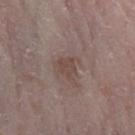Assessment:
Imaged during a routine full-body skin examination; the lesion was not biopsied and no histopathology is available.
Image and clinical context:
An algorithmic analysis of the crop reported an eccentricity of roughly 0.55 and a symmetry-axis asymmetry near 0.3. And it measured a lesion color around L≈45 a*≈15 b*≈21 in CIELAB. It also reported a border-irregularity index near 3/10, a within-lesion color-variation index near 1.5/10, and peripheral color asymmetry of about 0.5. The analysis additionally found an automated nevus-likeness rating near 10 out of 100 and a lesion-detection confidence of about 100/100. On the left forearm. Imaged with white-light lighting. Longest diameter approximately 2.5 mm. A female subject aged approximately 65. A roughly 15 mm field-of-view crop from a total-body skin photograph.